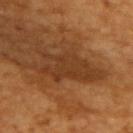<record>
<lesion_size>
  <long_diameter_mm_approx>7.5</long_diameter_mm_approx>
</lesion_size>
<patient>
  <sex>male</sex>
  <age_approx>65</age_approx>
</patient>
<image>
  <source>total-body photography crop</source>
  <field_of_view_mm>15</field_of_view_mm>
</image>
<automated_metrics>
  <area_mm2_approx>20.0</area_mm2_approx>
  <shape_asymmetry>0.45</shape_asymmetry>
  <vs_skin_darker_L>8.0</vs_skin_darker_L>
  <vs_skin_contrast_norm>7.0</vs_skin_contrast_norm>
</automated_metrics>
<lighting>cross-polarized</lighting>
</record>